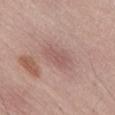Imaged during a routine full-body skin examination; the lesion was not biopsied and no histopathology is available.
A close-up tile cropped from a whole-body skin photograph, about 15 mm across.
Approximately 3 mm at its widest.
A male subject aged 53–57.
This is a white-light tile.